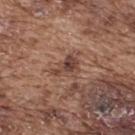Captured during whole-body skin photography for melanoma surveillance; the lesion was not biopsied.